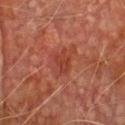Assessment:
Part of a total-body skin-imaging series; this lesion was reviewed on a skin check and was not flagged for biopsy.
Acquisition and patient details:
A roughly 15 mm field-of-view crop from a total-body skin photograph. On the chest. The subject is a male aged 73 to 77. The lesion's longest dimension is about 3 mm. The tile uses cross-polarized illumination. Automated image analysis of the tile measured a footprint of about 4.5 mm², an outline eccentricity of about 0.7 (0 = round, 1 = elongated), and a shape-asymmetry score of about 0.35 (0 = symmetric). The software also gave border irregularity of about 3.5 on a 0–10 scale, a within-lesion color-variation index near 3/10, and peripheral color asymmetry of about 1.5. And it measured a classifier nevus-likeness of about 0/100 and a detector confidence of about 100 out of 100 that the crop contains a lesion.